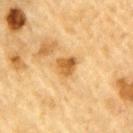{
  "site": "left upper arm",
  "lighting": "cross-polarized",
  "image": {
    "source": "total-body photography crop",
    "field_of_view_mm": 15
  },
  "patient": {
    "sex": "male",
    "age_approx": "85+"
  }
}